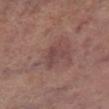biopsy status = total-body-photography surveillance lesion; no biopsy | image-analysis metrics = a footprint of about 4 mm² and a symmetry-axis asymmetry near 0.6; a mean CIELAB color near L≈43 a*≈20 b*≈20, a lesion–skin lightness drop of about 6, and a normalized border contrast of about 5.5; lesion-presence confidence of about 90/100 | acquisition = ~15 mm tile from a whole-body skin photo | subject = male, aged around 70 | size = ≈2.5 mm | lighting = white-light illumination | body site = the right lower leg.